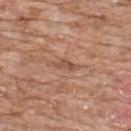Case summary:
* biopsy status · catalogued during a skin exam; not biopsied
* acquisition · 15 mm crop, total-body photography
* tile lighting · white-light illumination
* TBP lesion metrics · a nevus-likeness score of about 0/100 and a detector confidence of about 85 out of 100 that the crop contains a lesion
* subject · male, aged approximately 60
* lesion diameter · about 3 mm
* location · the chest